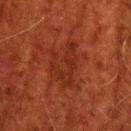Part of a total-body skin-imaging series; this lesion was reviewed on a skin check and was not flagged for biopsy. A male patient aged 58 to 62. The lesion is located on the head or neck. Cropped from a total-body skin-imaging series; the visible field is about 15 mm.